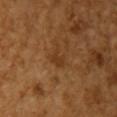biopsy status = imaged on a skin check; not biopsied
lighting = cross-polarized
body site = the arm
subject = female, in their mid-50s
imaging modality = ~15 mm tile from a whole-body skin photo